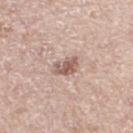{
  "biopsy_status": "not biopsied; imaged during a skin examination",
  "lighting": "white-light",
  "patient": {
    "sex": "male",
    "age_approx": 65
  },
  "image": {
    "source": "total-body photography crop",
    "field_of_view_mm": 15
  },
  "lesion_size": {
    "long_diameter_mm_approx": 3.0
  },
  "site": "leg"
}